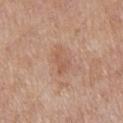The recorded lesion diameter is about 4 mm.
On the arm.
Cropped from a whole-body photographic skin survey; the tile spans about 15 mm.
The subject is a male aged around 55.
Imaged with white-light lighting.